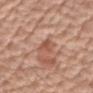The lesion was tiled from a total-body skin photograph and was not biopsied. A region of skin cropped from a whole-body photographic capture, roughly 15 mm wide. The lesion is located on the right upper arm. Measured at roughly 3 mm in maximum diameter. The total-body-photography lesion software estimated a footprint of about 4.5 mm², an eccentricity of roughly 0.8, and two-axis asymmetry of about 0.3. The analysis additionally found a lesion color around L≈54 a*≈24 b*≈29 in CIELAB and a lesion-to-skin contrast of about 6 (normalized; higher = more distinct). A male subject, aged 68 to 72. The tile uses white-light illumination.